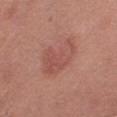The lesion was tiled from a total-body skin photograph and was not biopsied.
Automated tile analysis of the lesion measured a symmetry-axis asymmetry near 0.45. The analysis additionally found a classifier nevus-likeness of about 60/100.
Imaged with white-light lighting.
On the right thigh.
The lesion's longest dimension is about 5.5 mm.
A female subject aged approximately 40.
A 15 mm close-up extracted from a 3D total-body photography capture.